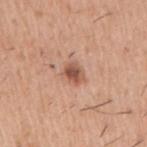Clinical impression: This lesion was catalogued during total-body skin photography and was not selected for biopsy. Acquisition and patient details: The lesion is located on the right upper arm. The total-body-photography lesion software estimated a normalized border contrast of about 8.5. It also reported a border-irregularity index near 2/10, a color-variation rating of about 4.5/10, and a peripheral color-asymmetry measure near 1.5. And it measured an automated nevus-likeness rating near 90 out of 100 and a lesion-detection confidence of about 100/100. This is a white-light tile. A 15 mm close-up tile from a total-body photography series done for melanoma screening. A male patient, about 40 years old. Measured at roughly 3 mm in maximum diameter.